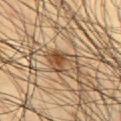Impression:
Part of a total-body skin-imaging series; this lesion was reviewed on a skin check and was not flagged for biopsy.
Context:
Captured under cross-polarized illumination. A 15 mm close-up tile from a total-body photography series done for melanoma screening. A male patient aged 58–62. The lesion is on the abdomen. The recorded lesion diameter is about 4 mm. Automated image analysis of the tile measured an outline eccentricity of about 0.7 (0 = round, 1 = elongated) and a symmetry-axis asymmetry near 0.45. And it measured a mean CIELAB color near L≈49 a*≈18 b*≈33, a lesion–skin lightness drop of about 12, and a normalized lesion–skin contrast near 9. It also reported a classifier nevus-likeness of about 75/100.